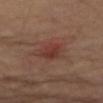Clinical impression:
The lesion was photographed on a routine skin check and not biopsied; there is no pathology result.
Context:
A subject in their mid- to late 50s. From the left upper arm. A 15 mm close-up extracted from a 3D total-body photography capture. Approximately 3 mm at its widest.